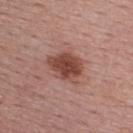Recorded during total-body skin imaging; not selected for excision or biopsy. The lesion-visualizer software estimated a shape eccentricity near 0.65 and a shape-asymmetry score of about 0.2 (0 = symmetric). And it measured a border-irregularity rating of about 2.5/10, a color-variation rating of about 3.5/10, and peripheral color asymmetry of about 1. And it measured an automated nevus-likeness rating near 90 out of 100 and a lesion-detection confidence of about 100/100. Located on the upper back. The tile uses white-light illumination. This image is a 15 mm lesion crop taken from a total-body photograph. The subject is a female roughly 55 years of age. The lesion's longest dimension is about 4.5 mm.